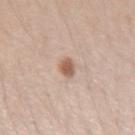This lesion was catalogued during total-body skin photography and was not selected for biopsy. The lesion is located on the arm. The total-body-photography lesion software estimated a lesion area of about 3 mm², a shape eccentricity near 0.55, and a symmetry-axis asymmetry near 0.15. The software also gave a border-irregularity rating of about 1/10 and internal color variation of about 2.5 on a 0–10 scale. It also reported a classifier nevus-likeness of about 95/100 and a lesion-detection confidence of about 100/100. A female subject in their mid- to late 20s. Cropped from a total-body skin-imaging series; the visible field is about 15 mm. This is a white-light tile.